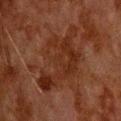notes — catalogued during a skin exam; not biopsied
diameter — ~9 mm (longest diameter)
acquisition — ~15 mm crop, total-body skin-cancer survey
lighting — cross-polarized
automated lesion analysis — a lesion–skin lightness drop of about 6 and a normalized lesion–skin contrast near 7
anatomic site — the upper back
patient — male, in their 80s